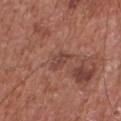<record>
  <biopsy_status>not biopsied; imaged during a skin examination</biopsy_status>
  <lesion_size>
    <long_diameter_mm_approx>3.0</long_diameter_mm_approx>
  </lesion_size>
  <lighting>white-light</lighting>
  <patient>
    <sex>male</sex>
    <age_approx>75</age_approx>
  </patient>
  <image>
    <source>total-body photography crop</source>
    <field_of_view_mm>15</field_of_view_mm>
  </image>
  <site>chest</site>
  <automated_metrics>
    <cielab_L>43</cielab_L>
    <cielab_a>23</cielab_a>
    <cielab_b>25</cielab_b>
    <vs_skin_darker_L>8.0</vs_skin_darker_L>
    <vs_skin_contrast_norm>6.5</vs_skin_contrast_norm>
    <border_irregularity_0_10>2.5</border_irregularity_0_10>
    <color_variation_0_10>2.5</color_variation_0_10>
    <peripheral_color_asymmetry>1.0</peripheral_color_asymmetry>
    <nevus_likeness_0_100>0</nevus_likeness_0_100>
    <lesion_detection_confidence_0_100>100</lesion_detection_confidence_0_100>
  </automated_metrics>
</record>